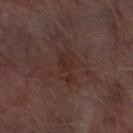Captured during whole-body skin photography for melanoma surveillance; the lesion was not biopsied.
The lesion's longest dimension is about 4 mm.
A region of skin cropped from a whole-body photographic capture, roughly 15 mm wide.
A male patient about 70 years old.
The tile uses white-light illumination.
The lesion is located on the arm.